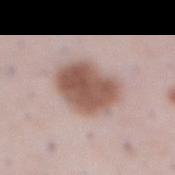Imaged with white-light lighting.
A 15 mm close-up tile from a total-body photography series done for melanoma screening.
The lesion is on the abdomen.
The subject is a male about 50 years old.